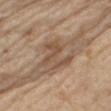notes: catalogued during a skin exam; not biopsied | image: total-body-photography crop, ~15 mm field of view | body site: the right thigh | patient: male, about 70 years old.